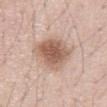Assessment:
This lesion was catalogued during total-body skin photography and was not selected for biopsy.
Clinical summary:
A 15 mm close-up tile from a total-body photography series done for melanoma screening. The lesion is located on the front of the torso. A male subject approximately 70 years of age.